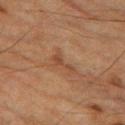{"biopsy_status": "not biopsied; imaged during a skin examination", "site": "left thigh", "patient": {"sex": "male", "age_approx": "85+"}, "lighting": "cross-polarized", "lesion_size": {"long_diameter_mm_approx": 3.0}, "automated_metrics": {"area_mm2_approx": 3.0, "eccentricity": 0.95, "shape_asymmetry": 0.55, "cielab_L": 39, "cielab_a": 19, "cielab_b": 29, "vs_skin_darker_L": 7.0, "vs_skin_contrast_norm": 6.0, "nevus_likeness_0_100": 0, "lesion_detection_confidence_0_100": 95}, "image": {"source": "total-body photography crop", "field_of_view_mm": 15}}